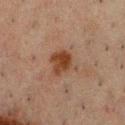Clinical impression:
This lesion was catalogued during total-body skin photography and was not selected for biopsy.
Clinical summary:
The lesion is on the chest. A male subject aged 48–52. This image is a 15 mm lesion crop taken from a total-body photograph.